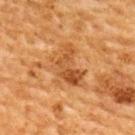No biopsy was performed on this lesion — it was imaged during a full skin examination and was not determined to be concerning. Imaged with cross-polarized lighting. A region of skin cropped from a whole-body photographic capture, roughly 15 mm wide. The lesion is located on the upper back. Automated image analysis of the tile measured a footprint of about 12 mm² and a shape-asymmetry score of about 0.4 (0 = symmetric). And it measured an average lesion color of about L≈44 a*≈22 b*≈37 (CIELAB), a lesion–skin lightness drop of about 8, and a normalized border contrast of about 7. And it measured border irregularity of about 5.5 on a 0–10 scale and a within-lesion color-variation index near 6/10. The analysis additionally found an automated nevus-likeness rating near 5 out of 100 and a detector confidence of about 100 out of 100 that the crop contains a lesion. The subject is a male about 60 years old. The recorded lesion diameter is about 5 mm.